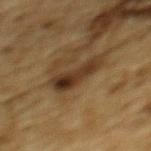Part of a total-body skin-imaging series; this lesion was reviewed on a skin check and was not flagged for biopsy. The patient is a male approximately 85 years of age or older. Automated image analysis of the tile measured a lesion color around L≈29 a*≈14 b*≈27 in CIELAB, about 10 CIELAB-L* units darker than the surrounding skin, and a normalized border contrast of about 10. The analysis additionally found a classifier nevus-likeness of about 25/100 and a lesion-detection confidence of about 100/100. Located on the upper back. Imaged with cross-polarized lighting. A lesion tile, about 15 mm wide, cut from a 3D total-body photograph. The lesion's longest dimension is about 5 mm.